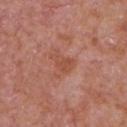Assessment: Imaged during a routine full-body skin examination; the lesion was not biopsied and no histopathology is available. Acquisition and patient details: A male subject aged approximately 65. A lesion tile, about 15 mm wide, cut from a 3D total-body photograph. On the front of the torso. Captured under white-light illumination.